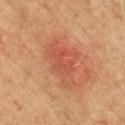Acquisition and patient details:
The tile uses cross-polarized illumination. The recorded lesion diameter is about 6.5 mm. A male subject, in their mid- to late 50s. The lesion is located on the chest. Cropped from a total-body skin-imaging series; the visible field is about 15 mm. Automated image analysis of the tile measured an average lesion color of about L≈47 a*≈27 b*≈31 (CIELAB), roughly 7 lightness units darker than nearby skin, and a lesion-to-skin contrast of about 5.5 (normalized; higher = more distinct). The analysis additionally found a border-irregularity index near 4.5/10, a within-lesion color-variation index near 4.5/10, and radial color variation of about 1.5. And it measured a classifier nevus-likeness of about 0/100.